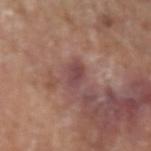Impression: Part of a total-body skin-imaging series; this lesion was reviewed on a skin check and was not flagged for biopsy. Image and clinical context: Measured at roughly 3.5 mm in maximum diameter. From the left upper arm. A male patient aged 78–82. Captured under white-light illumination. The total-body-photography lesion software estimated a lesion–skin lightness drop of about 9 and a lesion-to-skin contrast of about 7.5 (normalized; higher = more distinct). The software also gave a classifier nevus-likeness of about 0/100. A 15 mm crop from a total-body photograph taken for skin-cancer surveillance.